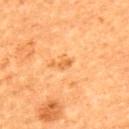  biopsy_status: not biopsied; imaged during a skin examination
  lighting: cross-polarized
  lesion_size:
    long_diameter_mm_approx: 2.0
  patient:
    sex: female
    age_approx: 55
  site: back
  image:
    source: total-body photography crop
    field_of_view_mm: 15